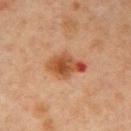Case summary:
• follow-up: total-body-photography surveillance lesion; no biopsy
• subject: male, about 45 years old
• anatomic site: the arm
• acquisition: total-body-photography crop, ~15 mm field of view